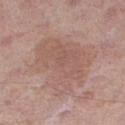workup = no biopsy performed (imaged during a skin exam); lesion diameter = ≈8 mm; body site = the right thigh; acquisition = 15 mm crop, total-body photography; lighting = white-light illumination; subject = male, aged 68–72.